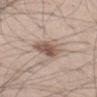biopsy status: catalogued during a skin exam; not biopsied
tile lighting: white-light illumination
TBP lesion metrics: a classifier nevus-likeness of about 90/100 and a detector confidence of about 100 out of 100 that the crop contains a lesion
imaging modality: 15 mm crop, total-body photography
body site: the left thigh
patient: male, approximately 30 years of age